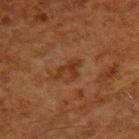Notes:
• follow-up: catalogued during a skin exam; not biopsied
• automated lesion analysis: a lesion area of about 4 mm², a shape eccentricity near 0.85, and two-axis asymmetry of about 0.6; an automated nevus-likeness rating near 0 out of 100 and a lesion-detection confidence of about 100/100
• lighting: cross-polarized
• anatomic site: the upper back
• image: ~15 mm tile from a whole-body skin photo
• subject: male, aged approximately 60
• size: ~3.5 mm (longest diameter)